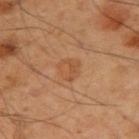Assessment: The lesion was tiled from a total-body skin photograph and was not biopsied. Background: The lesion-visualizer software estimated a footprint of about 5.5 mm², an eccentricity of roughly 0.45, and a symmetry-axis asymmetry near 0.25. This is a cross-polarized tile. A region of skin cropped from a whole-body photographic capture, roughly 15 mm wide. A male patient, aged approximately 50. Measured at roughly 2.5 mm in maximum diameter. The lesion is located on the left arm.